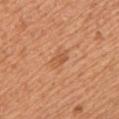notes: total-body-photography surveillance lesion; no biopsy | acquisition: ~15 mm tile from a whole-body skin photo | site: the left upper arm | lighting: white-light | subject: male, aged approximately 55 | image-analysis metrics: an area of roughly 3.5 mm², an eccentricity of roughly 0.8, and a shape-asymmetry score of about 0.25 (0 = symmetric); a lesion color around L≈57 a*≈25 b*≈39 in CIELAB, roughly 7 lightness units darker than nearby skin, and a normalized lesion–skin contrast near 5.5; internal color variation of about 1.5 on a 0–10 scale and peripheral color asymmetry of about 0.5; an automated nevus-likeness rating near 0 out of 100.